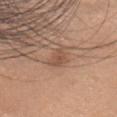No biopsy was performed on this lesion — it was imaged during a full skin examination and was not determined to be concerning. Automated image analysis of the tile measured an outline eccentricity of about 0.7 (0 = round, 1 = elongated) and a symmetry-axis asymmetry near 0.45. The software also gave a border-irregularity index near 4.5/10 and a within-lesion color-variation index near 1.5/10. The analysis additionally found an automated nevus-likeness rating near 30 out of 100 and a lesion-detection confidence of about 100/100. A 15 mm close-up tile from a total-body photography series done for melanoma screening. The tile uses white-light illumination. The patient is a female in their mid- to late 40s. About 3 mm across. Located on the head or neck.